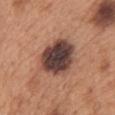{
  "biopsy_status": "not biopsied; imaged during a skin examination",
  "patient": {
    "sex": "male",
    "age_approx": 65
  },
  "image": {
    "source": "total-body photography crop",
    "field_of_view_mm": 15
  },
  "site": "chest",
  "lighting": "white-light"
}